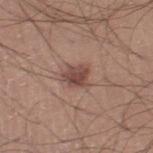<tbp_lesion>
  <image>
    <source>total-body photography crop</source>
    <field_of_view_mm>15</field_of_view_mm>
  </image>
  <automated_metrics>
    <area_mm2_approx>5.5</area_mm2_approx>
    <eccentricity>0.55</eccentricity>
    <shape_asymmetry>0.25</shape_asymmetry>
    <color_variation_0_10>3.5</color_variation_0_10>
  </automated_metrics>
  <lighting>white-light</lighting>
  <site>left thigh</site>
  <patient>
    <sex>male</sex>
    <age_approx>30</age_approx>
  </patient>
</tbp_lesion>